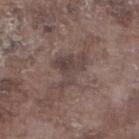Q: Was this lesion biopsied?
A: no biopsy performed (imaged during a skin exam)
Q: How was this image acquired?
A: ~15 mm tile from a whole-body skin photo
Q: Patient demographics?
A: male, about 75 years old
Q: What is the anatomic site?
A: the right lower leg
Q: What lighting was used for the tile?
A: white-light
Q: What did automated image analysis measure?
A: an average lesion color of about L≈42 a*≈14 b*≈18 (CIELAB) and a lesion-to-skin contrast of about 6.5 (normalized; higher = more distinct); a color-variation rating of about 4/10 and a peripheral color-asymmetry measure near 1.5; a classifier nevus-likeness of about 0/100 and lesion-presence confidence of about 95/100